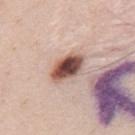Assessment:
The lesion was photographed on a routine skin check and not biopsied; there is no pathology result.
Clinical summary:
A male patient approximately 35 years of age. The lesion is located on the back. Cropped from a total-body skin-imaging series; the visible field is about 15 mm. This is a white-light tile. Longest diameter approximately 4.5 mm.